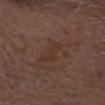Q: Is there a histopathology result?
A: imaged on a skin check; not biopsied